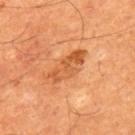The lesion was tiled from a total-body skin photograph and was not biopsied. A male patient, in their mid-50s. Cropped from a total-body skin-imaging series; the visible field is about 15 mm. The total-body-photography lesion software estimated a lesion area of about 9.5 mm² and a shape-asymmetry score of about 0.3 (0 = symmetric). It also reported a border-irregularity index near 4/10, a within-lesion color-variation index near 4.5/10, and a peripheral color-asymmetry measure near 1.5. The lesion is located on the back. Approximately 5.5 mm at its widest.